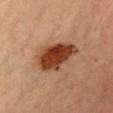workup: total-body-photography surveillance lesion; no biopsy
lesion diameter: ≈6 mm
imaging modality: total-body-photography crop, ~15 mm field of view
patient: female, aged 33 to 37
body site: the front of the torso
lighting: cross-polarized
image-analysis metrics: roughly 17 lightness units darker than nearby skin; a border-irregularity index near 2.5/10, a color-variation rating of about 5.5/10, and a peripheral color-asymmetry measure near 1.5; an automated nevus-likeness rating near 100 out of 100